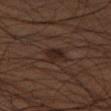Context:
A male subject aged 53 to 57. Captured under cross-polarized illumination. A close-up tile cropped from a whole-body skin photograph, about 15 mm across. The lesion is on the right thigh. Approximately 2.5 mm at its widest.